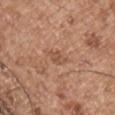  biopsy_status: not biopsied; imaged during a skin examination